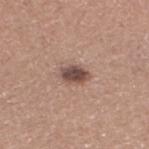  biopsy_status: not biopsied; imaged during a skin examination
  image:
    source: total-body photography crop
    field_of_view_mm: 15
  automated_metrics:
    cielab_L: 49
    cielab_a: 18
    cielab_b: 22
    vs_skin_darker_L: 14.0
    vs_skin_contrast_norm: 10.0
    border_irregularity_0_10: 2.0
    color_variation_0_10: 4.5
    peripheral_color_asymmetry: 1.5
  patient:
    sex: female
    age_approx: 35
  site: leg
  lighting: white-light